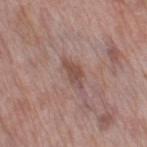Clinical summary: A female subject approximately 50 years of age. From the right thigh. A roughly 15 mm field-of-view crop from a total-body skin photograph.